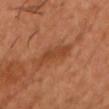size: ≈5.5 mm | lighting: cross-polarized illumination | body site: the chest | image source: ~15 mm crop, total-body skin-cancer survey | image-analysis metrics: an automated nevus-likeness rating near 35 out of 100 | patient: male, aged 38–42.